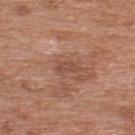Impression:
The lesion was photographed on a routine skin check and not biopsied; there is no pathology result.
Image and clinical context:
Automated tile analysis of the lesion measured a footprint of about 3 mm², an outline eccentricity of about 0.85 (0 = round, 1 = elongated), and a symmetry-axis asymmetry near 0.35. The software also gave a lesion color around L≈49 a*≈22 b*≈28 in CIELAB, about 8 CIELAB-L* units darker than the surrounding skin, and a lesion-to-skin contrast of about 6 (normalized; higher = more distinct). It also reported a border-irregularity index near 3.5/10, a color-variation rating of about 1.5/10, and peripheral color asymmetry of about 0.5. A 15 mm close-up extracted from a 3D total-body photography capture. A male patient aged around 70. The tile uses white-light illumination. The lesion is located on the upper back. Measured at roughly 2.5 mm in maximum diameter.